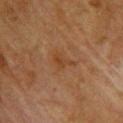biopsy status=no biopsy performed (imaged during a skin exam)
image source=~15 mm crop, total-body skin-cancer survey
anatomic site=the upper back
lesion size=≈2.5 mm
subject=female, aged 78–82
tile lighting=cross-polarized illumination